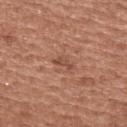The lesion was photographed on a routine skin check and not biopsied; there is no pathology result.
A male subject aged approximately 55.
The lesion is located on the upper back.
Imaged with white-light lighting.
The total-body-photography lesion software estimated a lesion–skin lightness drop of about 9 and a lesion-to-skin contrast of about 6.5 (normalized; higher = more distinct). And it measured a border-irregularity index near 5/10, a color-variation rating of about 0/10, and a peripheral color-asymmetry measure near 0. The software also gave a classifier nevus-likeness of about 0/100.
Longest diameter approximately 2.5 mm.
Cropped from a whole-body photographic skin survey; the tile spans about 15 mm.